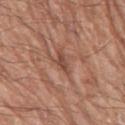follow-up: catalogued during a skin exam; not biopsied | acquisition: ~15 mm crop, total-body skin-cancer survey | lesion diameter: about 3 mm | subject: male, in their 70s | tile lighting: white-light illumination | body site: the leg | TBP lesion metrics: an automated nevus-likeness rating near 0 out of 100 and a detector confidence of about 55 out of 100 that the crop contains a lesion.